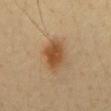notes: imaged on a skin check; not biopsied
illumination: cross-polarized illumination
image: 15 mm crop, total-body photography
diameter: ~4.5 mm (longest diameter)
subject: male, in their mid-40s
body site: the abdomen